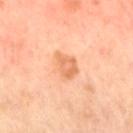Captured during whole-body skin photography for melanoma surveillance; the lesion was not biopsied.
The tile uses cross-polarized illumination.
A roughly 15 mm field-of-view crop from a total-body skin photograph.
Automated image analysis of the tile measured a lesion area of about 5.5 mm², an outline eccentricity of about 0.7 (0 = round, 1 = elongated), and two-axis asymmetry of about 0.3. The analysis additionally found a classifier nevus-likeness of about 5/100 and a lesion-detection confidence of about 100/100.
A male patient aged 63 to 67.
The lesion's longest dimension is about 3 mm.